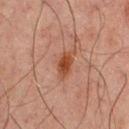Notes:
• follow-up: no biopsy performed (imaged during a skin exam)
• subject: male, roughly 65 years of age
• size: ~3.5 mm (longest diameter)
• image source: ~15 mm crop, total-body skin-cancer survey
• anatomic site: the upper back
• image-analysis metrics: a border-irregularity index near 2/10 and peripheral color asymmetry of about 0.5; an automated nevus-likeness rating near 95 out of 100